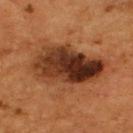workup: catalogued during a skin exam; not biopsied | subject: male, aged 53–57 | lesion size: ~8.5 mm (longest diameter) | automated metrics: a footprint of about 32 mm², an eccentricity of roughly 0.85, and a symmetry-axis asymmetry near 0.25 | location: the upper back | illumination: cross-polarized | image source: ~15 mm crop, total-body skin-cancer survey.